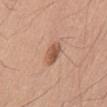Part of a total-body skin-imaging series; this lesion was reviewed on a skin check and was not flagged for biopsy.
Located on the front of the torso.
A male patient, aged around 60.
Longest diameter approximately 3 mm.
This image is a 15 mm lesion crop taken from a total-body photograph.
Imaged with white-light lighting.
The lesion-visualizer software estimated an area of roughly 5 mm², an eccentricity of roughly 0.7, and a symmetry-axis asymmetry near 0.2.